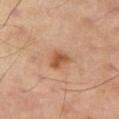Q: Is there a histopathology result?
A: catalogued during a skin exam; not biopsied
Q: What kind of image is this?
A: ~15 mm crop, total-body skin-cancer survey
Q: Automated lesion metrics?
A: a footprint of about 5 mm², a shape eccentricity near 0.7, and two-axis asymmetry of about 0.35; a lesion color around L≈55 a*≈23 b*≈36 in CIELAB and roughly 11 lightness units darker than nearby skin; a classifier nevus-likeness of about 80/100 and a lesion-detection confidence of about 100/100
Q: How was the tile lit?
A: cross-polarized
Q: Lesion location?
A: the right thigh